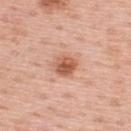follow-up: catalogued during a skin exam; not biopsied
body site: the upper back
patient: male, aged 58–62
imaging modality: total-body-photography crop, ~15 mm field of view